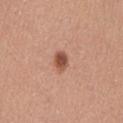Captured during whole-body skin photography for melanoma surveillance; the lesion was not biopsied. A lesion tile, about 15 mm wide, cut from a 3D total-body photograph. A female subject, in their 50s. The recorded lesion diameter is about 2.5 mm. Located on the abdomen. The tile uses white-light illumination.